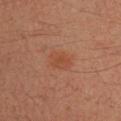Captured during whole-body skin photography for melanoma surveillance; the lesion was not biopsied. A 15 mm close-up extracted from a 3D total-body photography capture. A male subject roughly 35 years of age. On the right upper arm. The tile uses cross-polarized illumination.